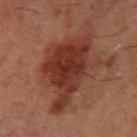Case summary:
– workup: no biopsy performed (imaged during a skin exam)
– lesion diameter: ≈8 mm
– image-analysis metrics: a footprint of about 31 mm², an outline eccentricity of about 0.75 (0 = round, 1 = elongated), and a symmetry-axis asymmetry near 0.4; a normalized border contrast of about 9.5; border irregularity of about 6 on a 0–10 scale and peripheral color asymmetry of about 1; a nevus-likeness score of about 0/100 and a lesion-detection confidence of about 100/100
– lighting: cross-polarized
– subject: male, aged 48 to 52
– image: ~15 mm tile from a whole-body skin photo
– body site: the left upper arm